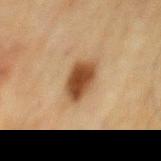Impression:
No biopsy was performed on this lesion — it was imaged during a full skin examination and was not determined to be concerning.
Background:
On the mid back. The patient is a male aged approximately 70. This image is a 15 mm lesion crop taken from a total-body photograph. The tile uses cross-polarized illumination.